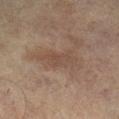Assessment: Imaged during a routine full-body skin examination; the lesion was not biopsied and no histopathology is available. Image and clinical context: Located on the right lower leg. This is a cross-polarized tile. A male subject, in their mid- to late 70s. This image is a 15 mm lesion crop taken from a total-body photograph.